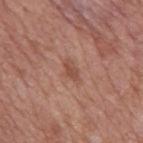workup: imaged on a skin check; not biopsied | anatomic site: the mid back | lesion diameter: ~3 mm (longest diameter) | imaging modality: total-body-photography crop, ~15 mm field of view | lighting: white-light illumination | patient: male, in their mid- to late 60s | image-analysis metrics: a shape eccentricity near 0.85 and a symmetry-axis asymmetry near 0.35; border irregularity of about 3.5 on a 0–10 scale.